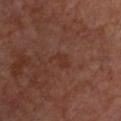The lesion was photographed on a routine skin check and not biopsied; there is no pathology result.
On the chest.
A female subject, in their 60s.
The tile uses cross-polarized illumination.
The lesion's longest dimension is about 2.5 mm.
A roughly 15 mm field-of-view crop from a total-body skin photograph.